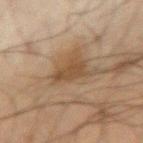{"biopsy_status": "not biopsied; imaged during a skin examination", "image": {"source": "total-body photography crop", "field_of_view_mm": 15}, "lesion_size": {"long_diameter_mm_approx": 3.5}, "site": "right forearm", "lighting": "cross-polarized", "automated_metrics": {"eccentricity": 0.65, "shape_asymmetry": 0.35, "border_irregularity_0_10": 3.5, "color_variation_0_10": 1.5, "peripheral_color_asymmetry": 0.5}, "patient": {"sex": "male", "age_approx": 70}}